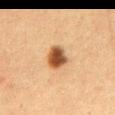<tbp_lesion>
  <biopsy_status>not biopsied; imaged during a skin examination</biopsy_status>
  <image>
    <source>total-body photography crop</source>
    <field_of_view_mm>15</field_of_view_mm>
  </image>
  <patient>
    <sex>male</sex>
    <age_approx>50</age_approx>
  </patient>
  <site>chest</site>
  <lighting>cross-polarized</lighting>
  <automated_metrics>
    <color_variation_0_10>5.0</color_variation_0_10>
    <peripheral_color_asymmetry>1.5</peripheral_color_asymmetry>
  </automated_metrics>
  <lesion_size>
    <long_diameter_mm_approx>3.5</long_diameter_mm_approx>
  </lesion_size>
</tbp_lesion>